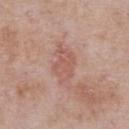{
  "image": {
    "source": "total-body photography crop",
    "field_of_view_mm": 15
  },
  "automated_metrics": {
    "area_mm2_approx": 8.0,
    "eccentricity": 0.8,
    "shape_asymmetry": 0.3,
    "nevus_likeness_0_100": 0,
    "lesion_detection_confidence_0_100": 100
  },
  "patient": {
    "sex": "male",
    "age_approx": 55
  },
  "site": "chest"
}